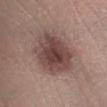This lesion was catalogued during total-body skin photography and was not selected for biopsy. This is a white-light tile. An algorithmic analysis of the crop reported about 12 CIELAB-L* units darker than the surrounding skin and a normalized lesion–skin contrast near 9.5. And it measured border irregularity of about 2.5 on a 0–10 scale and a within-lesion color-variation index near 5.5/10. The analysis additionally found a nevus-likeness score of about 45/100 and lesion-presence confidence of about 100/100. Located on the left lower leg. Cropped from a total-body skin-imaging series; the visible field is about 15 mm. A female subject, about 55 years old.